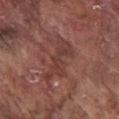- workup — no biopsy performed (imaged during a skin exam)
- automated lesion analysis — a border-irregularity index near 8.5/10
- acquisition — 15 mm crop, total-body photography
- tile lighting — white-light
- site — the chest
- lesion size — about 5.5 mm
- subject — male, roughly 75 years of age